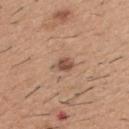<case>
<biopsy_status>not biopsied; imaged during a skin examination</biopsy_status>
<site>mid back</site>
<image>
  <source>total-body photography crop</source>
  <field_of_view_mm>15</field_of_view_mm>
</image>
<patient>
  <sex>male</sex>
  <age_approx>60</age_approx>
</patient>
<lighting>white-light</lighting>
<lesion_size>
  <long_diameter_mm_approx>3.0</long_diameter_mm_approx>
</lesion_size>
</case>